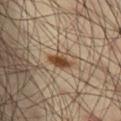biopsy status=imaged on a skin check; not biopsied
automated metrics=a lesion area of about 5 mm² and an eccentricity of roughly 0.75; an average lesion color of about L≈46 a*≈17 b*≈32 (CIELAB), a lesion–skin lightness drop of about 13, and a normalized border contrast of about 10; a within-lesion color-variation index near 4/10 and peripheral color asymmetry of about 1; an automated nevus-likeness rating near 90 out of 100
location=the left thigh
subject=male, aged approximately 40
lesion diameter=~3.5 mm (longest diameter)
acquisition=total-body-photography crop, ~15 mm field of view